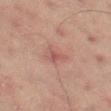No biopsy was performed on this lesion — it was imaged during a full skin examination and was not determined to be concerning.
A male subject, aged 58 to 62.
The lesion's longest dimension is about 3 mm.
The total-body-photography lesion software estimated an area of roughly 4.5 mm² and a shape eccentricity near 0.8. The analysis additionally found a lesion color around L≈48 a*≈21 b*≈22 in CIELAB and roughly 7 lightness units darker than nearby skin. And it measured a border-irregularity index near 5/10 and a peripheral color-asymmetry measure near 1.5. And it measured a classifier nevus-likeness of about 0/100.
The lesion is on the left thigh.
Cropped from a whole-body photographic skin survey; the tile spans about 15 mm.
The tile uses cross-polarized illumination.